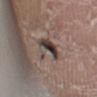Findings:
* size: ≈4 mm
* patient: female, approximately 70 years of age
* acquisition: ~15 mm crop, total-body skin-cancer survey
* site: the right lower leg
* illumination: white-light illumination